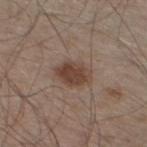{"biopsy_status": "not biopsied; imaged during a skin examination", "image": {"source": "total-body photography crop", "field_of_view_mm": 15}, "patient": {"sex": "male", "age_approx": 60}, "site": "left lower leg"}